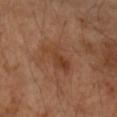Impression: This lesion was catalogued during total-body skin photography and was not selected for biopsy. Background: This is a cross-polarized tile. Cropped from a total-body skin-imaging series; the visible field is about 15 mm. From the right forearm. The patient is a female aged around 60. Automated image analysis of the tile measured a lesion area of about 8.5 mm², a shape eccentricity near 0.9, and a shape-asymmetry score of about 0.4 (0 = symmetric). The software also gave a mean CIELAB color near L≈42 a*≈22 b*≈31, about 7 CIELAB-L* units darker than the surrounding skin, and a normalized lesion–skin contrast near 6. The software also gave a classifier nevus-likeness of about 0/100 and a detector confidence of about 100 out of 100 that the crop contains a lesion.